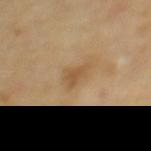* size · ≈3 mm
* image · ~15 mm tile from a whole-body skin photo
* site · the upper back
* illumination · cross-polarized
* automated lesion analysis · an area of roughly 4.5 mm², an eccentricity of roughly 0.75, and two-axis asymmetry of about 0.45; a border-irregularity index near 4.5/10, internal color variation of about 1.5 on a 0–10 scale, and peripheral color asymmetry of about 0.5
* subject · female, aged approximately 70